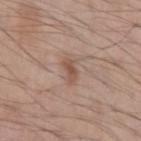Recorded during total-body skin imaging; not selected for excision or biopsy. Approximately 2.5 mm at its widest. A region of skin cropped from a whole-body photographic capture, roughly 15 mm wide. Located on the left upper arm. This is a white-light tile. A male patient roughly 70 years of age.